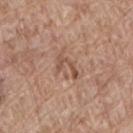Recorded during total-body skin imaging; not selected for excision or biopsy.